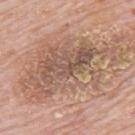Imaged during a routine full-body skin examination; the lesion was not biopsied and no histopathology is available. The tile uses white-light illumination. A male subject, roughly 80 years of age. From the upper back. A roughly 15 mm field-of-view crop from a total-body skin photograph. Automated image analysis of the tile measured an outline eccentricity of about 0.85 (0 = round, 1 = elongated) and a shape-asymmetry score of about 0.4 (0 = symmetric). It also reported a lesion–skin lightness drop of about 10. Approximately 12 mm at its widest.